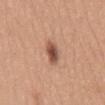Located on the mid back. A female subject, aged 28–32. Cropped from a whole-body photographic skin survey; the tile spans about 15 mm. The recorded lesion diameter is about 3 mm.